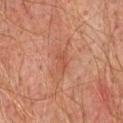  biopsy_status: not biopsied; imaged during a skin examination
  patient:
    sex: male
    age_approx: 75
  image:
    source: total-body photography crop
    field_of_view_mm: 15
  site: chest